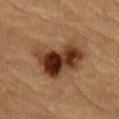Captured during whole-body skin photography for melanoma surveillance; the lesion was not biopsied. A male subject, in their mid- to late 80s or older. A region of skin cropped from a whole-body photographic capture, roughly 15 mm wide. Imaged with cross-polarized lighting. Longest diameter approximately 6.5 mm. The lesion is located on the abdomen. The lesion-visualizer software estimated a color-variation rating of about 10/10 and radial color variation of about 4. It also reported a classifier nevus-likeness of about 95/100 and a detector confidence of about 100 out of 100 that the crop contains a lesion.